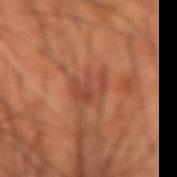Part of a total-body skin-imaging series; this lesion was reviewed on a skin check and was not flagged for biopsy. From the right forearm. A 15 mm close-up tile from a total-body photography series done for melanoma screening. Automated image analysis of the tile measured a footprint of about 6.5 mm², an eccentricity of roughly 0.65, and a symmetry-axis asymmetry near 0.35. The analysis additionally found border irregularity of about 4.5 on a 0–10 scale, a color-variation rating of about 4/10, and a peripheral color-asymmetry measure near 1.5. And it measured an automated nevus-likeness rating near 0 out of 100 and lesion-presence confidence of about 90/100. The patient is a male roughly 55 years of age. This is a cross-polarized tile.